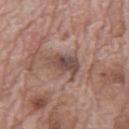{"lesion_size": {"long_diameter_mm_approx": 4.0}, "image": {"source": "total-body photography crop", "field_of_view_mm": 15}, "patient": {"sex": "male", "age_approx": 70}, "site": "back", "lighting": "white-light"}